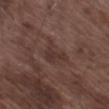  biopsy_status: not biopsied; imaged during a skin examination